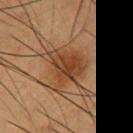Notes:
* follow-up — total-body-photography surveillance lesion; no biopsy
* automated metrics — a footprint of about 15 mm² and a symmetry-axis asymmetry near 0.35; a detector confidence of about 100 out of 100 that the crop contains a lesion
* image source — total-body-photography crop, ~15 mm field of view
* body site — the arm
* tile lighting — cross-polarized illumination
* lesion size — ~5 mm (longest diameter)
* patient — male, aged 53 to 57